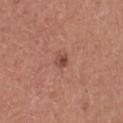biopsy_status: not biopsied; imaged during a skin examination
patient:
  sex: female
  age_approx: 25
site: chest
image:
  source: total-body photography crop
  field_of_view_mm: 15